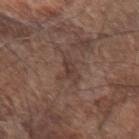Recorded during total-body skin imaging; not selected for excision or biopsy.
The lesion is on the left forearm.
The patient is a male in their 70s.
Approximately 4 mm at its widest.
This is a white-light tile.
Cropped from a whole-body photographic skin survey; the tile spans about 15 mm.